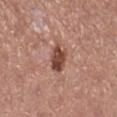notes: imaged on a skin check; not biopsied
image source: total-body-photography crop, ~15 mm field of view
location: the leg
patient: female, roughly 60 years of age
lesion size: ~3.5 mm (longest diameter)
automated metrics: lesion-presence confidence of about 100/100